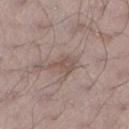No biopsy was performed on this lesion — it was imaged during a full skin examination and was not determined to be concerning.
The patient is a male aged 23 to 27.
The recorded lesion diameter is about 4 mm.
This is a white-light tile.
The total-body-photography lesion software estimated internal color variation of about 2.5 on a 0–10 scale and radial color variation of about 1.
Located on the left lower leg.
A roughly 15 mm field-of-view crop from a total-body skin photograph.